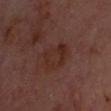On the head or neck. An algorithmic analysis of the crop reported an area of roughly 7.5 mm², an eccentricity of roughly 0.85, and two-axis asymmetry of about 0.35. And it measured a lesion color around L≈27 a*≈20 b*≈23 in CIELAB and a lesion-to-skin contrast of about 6.5 (normalized; higher = more distinct). It also reported a border-irregularity index near 7/10, a within-lesion color-variation index near 2/10, and peripheral color asymmetry of about 0.5. The analysis additionally found an automated nevus-likeness rating near 15 out of 100 and lesion-presence confidence of about 100/100. A subject aged 63–67. A 15 mm close-up extracted from a 3D total-body photography capture. Captured under cross-polarized illumination.